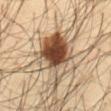tile lighting: cross-polarized
patient: male, aged approximately 35
site: the abdomen
automated metrics: an area of roughly 22 mm², an eccentricity of roughly 0.7, and a symmetry-axis asymmetry near 0.3; a lesion color around L≈46 a*≈15 b*≈29 in CIELAB and a lesion-to-skin contrast of about 12 (normalized; higher = more distinct); a border-irregularity index near 4.5/10, a within-lesion color-variation index near 10/10, and a peripheral color-asymmetry measure near 5; a nevus-likeness score of about 100/100
acquisition: 15 mm crop, total-body photography